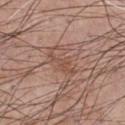<record>
  <biopsy_status>not biopsied; imaged during a skin examination</biopsy_status>
  <automated_metrics>
    <eccentricity>0.8</eccentricity>
    <shape_asymmetry>0.6</shape_asymmetry>
    <color_variation_0_10>2.0</color_variation_0_10>
    <peripheral_color_asymmetry>0.5</peripheral_color_asymmetry>
    <lesion_detection_confidence_0_100>100</lesion_detection_confidence_0_100>
  </automated_metrics>
  <patient>
    <sex>male</sex>
    <age_approx>55</age_approx>
  </patient>
  <site>chest</site>
  <lesion_size>
    <long_diameter_mm_approx>4.5</long_diameter_mm_approx>
  </lesion_size>
  <lighting>white-light</lighting>
  <image>
    <source>total-body photography crop</source>
    <field_of_view_mm>15</field_of_view_mm>
  </image>
</record>